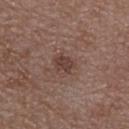Q: Was this lesion biopsied?
A: total-body-photography surveillance lesion; no biopsy
Q: What did automated image analysis measure?
A: a mean CIELAB color near L≈39 a*≈18 b*≈22 and a normalized border contrast of about 7.5
Q: What is the imaging modality?
A: 15 mm crop, total-body photography
Q: Who is the patient?
A: female, in their mid-60s
Q: Lesion size?
A: ~3 mm (longest diameter)
Q: Where on the body is the lesion?
A: the upper back
Q: How was the tile lit?
A: white-light